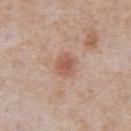follow-up: total-body-photography surveillance lesion; no biopsy
imaging modality: ~15 mm tile from a whole-body skin photo
automated metrics: a lesion area of about 5.5 mm²; a border-irregularity index near 2/10, a within-lesion color-variation index near 2/10, and peripheral color asymmetry of about 0.5; a classifier nevus-likeness of about 65/100 and lesion-presence confidence of about 100/100
lighting: white-light
subject: male, aged approximately 65
lesion diameter: about 2.5 mm
location: the abdomen